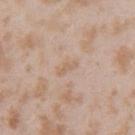The lesion was tiled from a total-body skin photograph and was not biopsied.
A female patient, in their mid- to late 20s.
The lesion-visualizer software estimated an area of roughly 3 mm², a shape eccentricity near 0.85, and two-axis asymmetry of about 0.35. It also reported an average lesion color of about L≈63 a*≈16 b*≈29 (CIELAB), roughly 6 lightness units darker than nearby skin, and a lesion-to-skin contrast of about 4.5 (normalized; higher = more distinct).
A region of skin cropped from a whole-body photographic capture, roughly 15 mm wide.
The lesion is located on the left upper arm.
The recorded lesion diameter is about 2.5 mm.